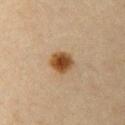The patient is a female aged around 45. Measured at roughly 3 mm in maximum diameter. A close-up tile cropped from a whole-body skin photograph, about 15 mm across. The lesion is located on the arm. Automated image analysis of the tile measured a footprint of about 6.5 mm² and a shape-asymmetry score of about 0.2 (0 = symmetric). The software also gave a mean CIELAB color near L≈42 a*≈18 b*≈33, a lesion–skin lightness drop of about 14, and a normalized lesion–skin contrast near 11.5. Imaged with cross-polarized lighting.